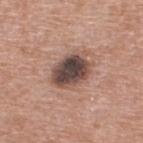Captured during whole-body skin photography for melanoma surveillance; the lesion was not biopsied. The lesion is located on the upper back. A 15 mm close-up extracted from a 3D total-body photography capture. Captured under white-light illumination. About 5 mm across. The patient is a male in their 70s.